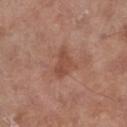Approximately 3.5 mm at its widest.
The lesion is located on the left lower leg.
The subject is a male aged 68 to 72.
This is a white-light tile.
A region of skin cropped from a whole-body photographic capture, roughly 15 mm wide.